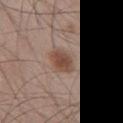image source = ~15 mm crop, total-body skin-cancer survey; subject = male, approximately 45 years of age; site = the left thigh.